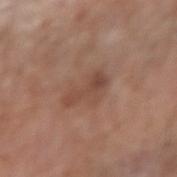Findings:
* subject · male, about 75 years old
* lesion diameter · ≈4 mm
* image source · ~15 mm tile from a whole-body skin photo
* body site · the left forearm